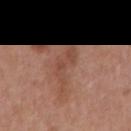Part of a total-body skin-imaging series; this lesion was reviewed on a skin check and was not flagged for biopsy. Captured under white-light illumination. An algorithmic analysis of the crop reported a mean CIELAB color near L≈47 a*≈22 b*≈28, roughly 7 lightness units darker than nearby skin, and a normalized lesion–skin contrast near 5.5. From the mid back. A region of skin cropped from a whole-body photographic capture, roughly 15 mm wide. The patient is a male aged around 70.